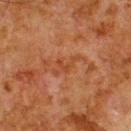The lesion was tiled from a total-body skin photograph and was not biopsied.
A 15 mm crop from a total-body photograph taken for skin-cancer surveillance.
On the upper back.
Approximately 4 mm at its widest.
A male patient aged 78–82.